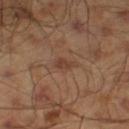Recorded during total-body skin imaging; not selected for excision or biopsy. The total-body-photography lesion software estimated an outline eccentricity of about 0.7 (0 = round, 1 = elongated) and two-axis asymmetry of about 0.25. The software also gave a border-irregularity rating of about 2.5/10, a within-lesion color-variation index near 2/10, and peripheral color asymmetry of about 0.5. The software also gave a classifier nevus-likeness of about 0/100. About 2.5 mm across. A male subject aged around 45. A region of skin cropped from a whole-body photographic capture, roughly 15 mm wide. The tile uses cross-polarized illumination. The lesion is located on the left lower leg.